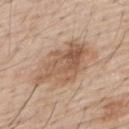Findings:
- workup: catalogued during a skin exam; not biopsied
- site: the upper back
- patient: male, aged 53–57
- lesion size: ~7.5 mm (longest diameter)
- image-analysis metrics: a border-irregularity rating of about 4.5/10, a within-lesion color-variation index near 6/10, and radial color variation of about 2.5
- image: ~15 mm crop, total-body skin-cancer survey